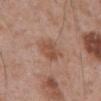| key | value |
|---|---|
| imaging modality | total-body-photography crop, ~15 mm field of view |
| lighting | white-light illumination |
| lesion size | ≈3 mm |
| automated metrics | a lesion color around L≈51 a*≈21 b*≈30 in CIELAB and a normalized border contrast of about 6.5 |
| patient | male, aged 68 to 72 |
| location | the abdomen |